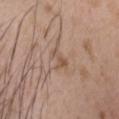Clinical impression:
No biopsy was performed on this lesion — it was imaged during a full skin examination and was not determined to be concerning.
Clinical summary:
Located on the head or neck. Approximately 2.5 mm at its widest. The subject is a male aged 23 to 27. A 15 mm close-up extracted from a 3D total-body photography capture.